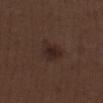No biopsy was performed on this lesion — it was imaged during a full skin examination and was not determined to be concerning. A male subject aged around 70. The lesion-visualizer software estimated a lesion color around L≈24 a*≈15 b*≈19 in CIELAB and about 6 CIELAB-L* units darker than the surrounding skin. It also reported a within-lesion color-variation index near 3.5/10. About 3 mm across. This image is a 15 mm lesion crop taken from a total-body photograph. On the right lower leg.